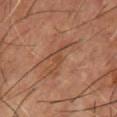Clinical summary: Approximately 6 mm at its widest. Captured under cross-polarized illumination. An algorithmic analysis of the crop reported a footprint of about 8 mm², a shape eccentricity near 0.95, and a shape-asymmetry score of about 0.5 (0 = symmetric). It also reported a lesion-to-skin contrast of about 5.5 (normalized; higher = more distinct). From the chest. A male subject aged approximately 55. A 15 mm close-up tile from a total-body photography series done for melanoma screening.